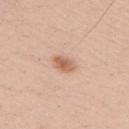The lesion was photographed on a routine skin check and not biopsied; there is no pathology result. From the upper back. Cropped from a total-body skin-imaging series; the visible field is about 15 mm. A male patient, aged 33 to 37.